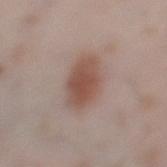{"biopsy_status": "not biopsied; imaged during a skin examination", "automated_metrics": {"eccentricity": 0.7, "shape_asymmetry": 0.15, "border_irregularity_0_10": 1.5, "color_variation_0_10": 3.0}, "lighting": "white-light", "image": {"source": "total-body photography crop", "field_of_view_mm": 15}, "lesion_size": {"long_diameter_mm_approx": 5.0}, "site": "left lower leg", "patient": {"sex": "female", "age_approx": 30}}